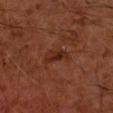Case summary:
* notes — total-body-photography surveillance lesion; no biopsy
* image — ~15 mm crop, total-body skin-cancer survey
* anatomic site — the arm
* tile lighting — cross-polarized illumination
* patient — male, aged 58–62